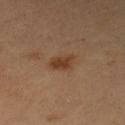Assessment:
This lesion was catalogued during total-body skin photography and was not selected for biopsy.
Acquisition and patient details:
Automated image analysis of the tile measured a lesion color around L≈41 a*≈20 b*≈32 in CIELAB and a lesion–skin lightness drop of about 9. A female subject, aged around 60. Imaged with cross-polarized lighting. The lesion is on the left lower leg. The lesion's longest dimension is about 3.5 mm. A lesion tile, about 15 mm wide, cut from a 3D total-body photograph.